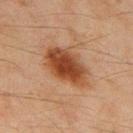Captured during whole-body skin photography for melanoma surveillance; the lesion was not biopsied. A roughly 15 mm field-of-view crop from a total-body skin photograph. Captured under cross-polarized illumination. A male patient, aged 43 to 47. Automated image analysis of the tile measured an automated nevus-likeness rating near 100 out of 100 and a lesion-detection confidence of about 100/100. The lesion is located on the upper back.